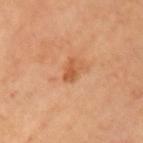Part of a total-body skin-imaging series; this lesion was reviewed on a skin check and was not flagged for biopsy. A lesion tile, about 15 mm wide, cut from a 3D total-body photograph. The lesion is located on the left upper arm. A female subject about 70 years old.